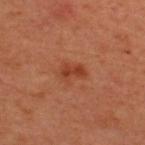Q: Is there a histopathology result?
A: catalogued during a skin exam; not biopsied
Q: What did automated image analysis measure?
A: a mean CIELAB color near L≈42 a*≈29 b*≈35, a lesion–skin lightness drop of about 8, and a normalized border contrast of about 7; a border-irregularity index near 3.5/10, a color-variation rating of about 2/10, and radial color variation of about 0.5
Q: How was this image acquired?
A: ~15 mm tile from a whole-body skin photo
Q: Who is the patient?
A: male, roughly 50 years of age
Q: Illumination type?
A: cross-polarized illumination
Q: Lesion location?
A: the upper back